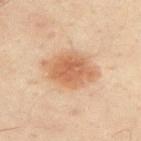Imaged during a routine full-body skin examination; the lesion was not biopsied and no histopathology is available. About 6.5 mm across. Automated tile analysis of the lesion measured internal color variation of about 3.5 on a 0–10 scale. From the upper back. Cropped from a total-body skin-imaging series; the visible field is about 15 mm. Imaged with cross-polarized lighting. A male subject aged 48–52.